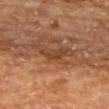{
  "biopsy_status": "not biopsied; imaged during a skin examination",
  "patient": {
    "sex": "male",
    "age_approx": 65
  },
  "site": "upper back",
  "lighting": "cross-polarized",
  "image": {
    "source": "total-body photography crop",
    "field_of_view_mm": 15
  }
}